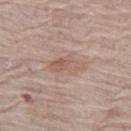The lesion was photographed on a routine skin check and not biopsied; there is no pathology result. A female subject aged approximately 65. Captured under white-light illumination. Longest diameter approximately 4 mm. Cropped from a total-body skin-imaging series; the visible field is about 15 mm. On the left thigh.